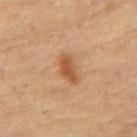Assessment:
Imaged during a routine full-body skin examination; the lesion was not biopsied and no histopathology is available.
Acquisition and patient details:
About 3.5 mm across. A male patient, in their mid- to late 70s. From the mid back. A lesion tile, about 15 mm wide, cut from a 3D total-body photograph. Captured under cross-polarized illumination.